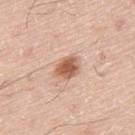Q: Was a biopsy performed?
A: imaged on a skin check; not biopsied
Q: What is the imaging modality?
A: ~15 mm crop, total-body skin-cancer survey
Q: What is the anatomic site?
A: the upper back
Q: Patient demographics?
A: male, aged around 75